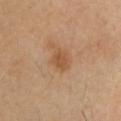Context: A male subject about 55 years old. From the head or neck. A close-up tile cropped from a whole-body skin photograph, about 15 mm across. Imaged with cross-polarized lighting. The lesion-visualizer software estimated a lesion area of about 4.5 mm² and two-axis asymmetry of about 0.25. The analysis additionally found an average lesion color of about L≈52 a*≈21 b*≈36 (CIELAB), roughly 8 lightness units darker than nearby skin, and a lesion-to-skin contrast of about 6.5 (normalized; higher = more distinct). The software also gave an automated nevus-likeness rating near 50 out of 100 and a detector confidence of about 100 out of 100 that the crop contains a lesion.